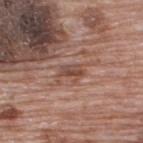Context: A male subject, approximately 70 years of age. Cropped from a whole-body photographic skin survey; the tile spans about 15 mm. The lesion-visualizer software estimated a lesion–skin lightness drop of about 9. The software also gave peripheral color asymmetry of about 1.5. And it measured a nevus-likeness score of about 0/100 and a lesion-detection confidence of about 90/100. Imaged with white-light lighting. The lesion is on the back. The lesion's longest dimension is about 2.5 mm.